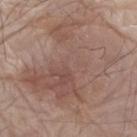Imaged during a routine full-body skin examination; the lesion was not biopsied and no histopathology is available.
Longest diameter approximately 10 mm.
The lesion-visualizer software estimated a lesion area of about 55 mm². The software also gave a nevus-likeness score of about 0/100 and lesion-presence confidence of about 100/100.
A male patient, about 80 years old.
A lesion tile, about 15 mm wide, cut from a 3D total-body photograph.
Located on the left arm.
The tile uses white-light illumination.